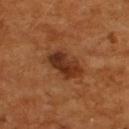This lesion was catalogued during total-body skin photography and was not selected for biopsy. On the back. Automated tile analysis of the lesion measured a lesion color around L≈31 a*≈22 b*≈30 in CIELAB, a lesion–skin lightness drop of about 10, and a lesion-to-skin contrast of about 9.5 (normalized; higher = more distinct). And it measured a lesion-detection confidence of about 100/100. Imaged with cross-polarized lighting. About 4.5 mm across. A male subject aged 53–57. A close-up tile cropped from a whole-body skin photograph, about 15 mm across.